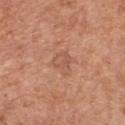follow-up = imaged on a skin check; not biopsied
patient = male, in their mid- to late 60s
diameter = ≈3 mm
location = the front of the torso
TBP lesion metrics = an automated nevus-likeness rating near 0 out of 100 and a detector confidence of about 100 out of 100 that the crop contains a lesion
image = total-body-photography crop, ~15 mm field of view
illumination = white-light illumination